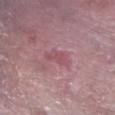The lesion was photographed on a routine skin check and not biopsied; there is no pathology result. A male subject aged 58 to 62. Measured at roughly 3.5 mm in maximum diameter. A 15 mm crop from a total-body photograph taken for skin-cancer surveillance. Captured under white-light illumination. The lesion is on the right forearm. Automated image analysis of the tile measured a mean CIELAB color near L≈51 a*≈27 b*≈16, roughly 6 lightness units darker than nearby skin, and a lesion-to-skin contrast of about 4.5 (normalized; higher = more distinct). The analysis additionally found a border-irregularity rating of about 4/10 and a within-lesion color-variation index near 1/10. And it measured a nevus-likeness score of about 0/100 and a lesion-detection confidence of about 60/100.